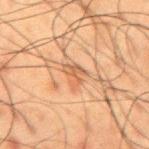workup: catalogued during a skin exam; not biopsied
patient: male, aged around 60
image source: ~15 mm tile from a whole-body skin photo
anatomic site: the mid back